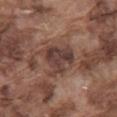Recorded during total-body skin imaging; not selected for excision or biopsy. The lesion is on the mid back. A male subject in their mid- to late 70s. The tile uses white-light illumination. A region of skin cropped from a whole-body photographic capture, roughly 15 mm wide. The total-body-photography lesion software estimated a shape eccentricity near 0.45 and a symmetry-axis asymmetry near 0.4. It also reported a nevus-likeness score of about 0/100.